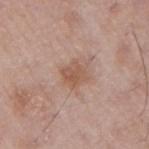Part of a total-body skin-imaging series; this lesion was reviewed on a skin check and was not flagged for biopsy. The tile uses white-light illumination. Longest diameter approximately 3 mm. The lesion is on the right upper arm. This image is a 15 mm lesion crop taken from a total-body photograph. A male patient, in their mid- to late 60s.